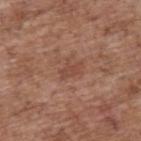biopsy_status: not biopsied; imaged during a skin examination
lighting: white-light
patient:
  sex: male
  age_approx: 70
automated_metrics:
  eccentricity: 0.8
  shape_asymmetry: 0.4
  border_irregularity_0_10: 4.5
  peripheral_color_asymmetry: 1.0
  nevus_likeness_0_100: 0
  lesion_detection_confidence_0_100: 100
lesion_size:
  long_diameter_mm_approx: 3.0
image:
  source: total-body photography crop
  field_of_view_mm: 15
site: upper back